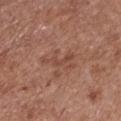| key | value |
|---|---|
| biopsy status | catalogued during a skin exam; not biopsied |
| TBP lesion metrics | a lesion area of about 6 mm², a shape eccentricity near 0.9, and a symmetry-axis asymmetry near 0.6; a border-irregularity index near 8.5/10 and internal color variation of about 2 on a 0–10 scale |
| lesion diameter | about 4.5 mm |
| location | the chest |
| imaging modality | ~15 mm tile from a whole-body skin photo |
| subject | female, aged around 75 |